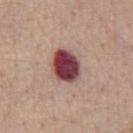No biopsy was performed on this lesion — it was imaged during a full skin examination and was not determined to be concerning. Captured under white-light illumination. The subject is a male aged 53 to 57. This image is a 15 mm lesion crop taken from a total-body photograph. On the chest. Automated image analysis of the tile measured an outline eccentricity of about 0.6 (0 = round, 1 = elongated) and a symmetry-axis asymmetry near 0.1. It also reported an average lesion color of about L≈43 a*≈27 b*≈18 (CIELAB), a lesion–skin lightness drop of about 22, and a normalized lesion–skin contrast near 15.5. And it measured internal color variation of about 7 on a 0–10 scale and peripheral color asymmetry of about 2. And it measured a classifier nevus-likeness of about 0/100 and a lesion-detection confidence of about 100/100. The recorded lesion diameter is about 4 mm.